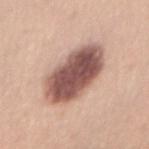| key | value |
|---|---|
| notes | no biopsy performed (imaged during a skin exam) |
| anatomic site | the abdomen |
| patient | female, aged 23–27 |
| illumination | white-light |
| diameter | about 7 mm |
| acquisition | 15 mm crop, total-body photography |